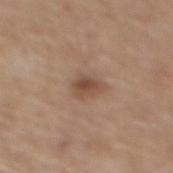The lesion was photographed on a routine skin check and not biopsied; there is no pathology result.
The total-body-photography lesion software estimated a mean CIELAB color near L≈48 a*≈18 b*≈28 and roughly 10 lightness units darker than nearby skin. It also reported a border-irregularity rating of about 2.5/10 and a color-variation rating of about 3/10. And it measured an automated nevus-likeness rating near 70 out of 100 and a detector confidence of about 100 out of 100 that the crop contains a lesion.
The lesion is located on the back.
A roughly 15 mm field-of-view crop from a total-body skin photograph.
About 2.5 mm across.
A male patient aged around 70.